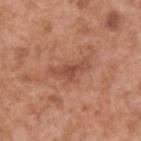• notes: catalogued during a skin exam; not biopsied
• subject: male, aged approximately 65
• location: the upper back
• imaging modality: ~15 mm tile from a whole-body skin photo